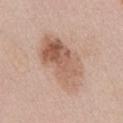Notes:
• location — the abdomen
• patient — male, aged 53 to 57
• acquisition — ~15 mm crop, total-body skin-cancer survey
• automated metrics — a lesion–skin lightness drop of about 11 and a normalized lesion–skin contrast near 7; a border-irregularity index near 3/10 and internal color variation of about 8 on a 0–10 scale